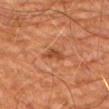tile lighting: cross-polarized | patient: male, aged approximately 65 | lesion size: ~3.5 mm (longest diameter) | image source: ~15 mm tile from a whole-body skin photo | body site: the left upper arm | TBP lesion metrics: an automated nevus-likeness rating near 10 out of 100.